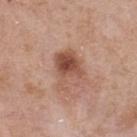Part of a total-body skin-imaging series; this lesion was reviewed on a skin check and was not flagged for biopsy. A male patient in their mid- to late 50s. Automated image analysis of the tile measured a mean CIELAB color near L≈52 a*≈22 b*≈29, a lesion–skin lightness drop of about 12, and a normalized border contrast of about 8. It also reported lesion-presence confidence of about 100/100. A 15 mm close-up tile from a total-body photography series done for melanoma screening. The tile uses white-light illumination. The lesion is on the upper back. The recorded lesion diameter is about 5 mm.